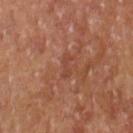Assessment:
The lesion was tiled from a total-body skin photograph and was not biopsied.
Acquisition and patient details:
The lesion-visualizer software estimated an average lesion color of about L≈42 a*≈24 b*≈29 (CIELAB), a lesion–skin lightness drop of about 5, and a normalized lesion–skin contrast near 4.5. And it measured a border-irregularity rating of about 6.5/10 and a color-variation rating of about 0/10. The software also gave an automated nevus-likeness rating near 0 out of 100 and lesion-presence confidence of about 95/100. Cropped from a whole-body photographic skin survey; the tile spans about 15 mm. A female patient approximately 70 years of age. This is a cross-polarized tile. The lesion is located on the left forearm.